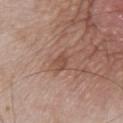• biopsy status · no biopsy performed (imaged during a skin exam)